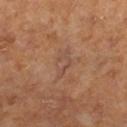Findings:
• site: the right lower leg
• acquisition: 15 mm crop, total-body photography
• patient: female, aged 63–67